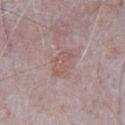<case>
<biopsy_status>not biopsied; imaged during a skin examination</biopsy_status>
<image>
  <source>total-body photography crop</source>
  <field_of_view_mm>15</field_of_view_mm>
</image>
<patient>
  <sex>male</sex>
  <age_approx>65</age_approx>
</patient>
<lighting>white-light</lighting>
<lesion_size>
  <long_diameter_mm_approx>4.0</long_diameter_mm_approx>
</lesion_size>
<site>chest</site>
</case>